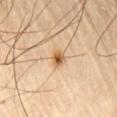Q: Is there a histopathology result?
A: imaged on a skin check; not biopsied
Q: What did automated image analysis measure?
A: an area of roughly 3 mm², an outline eccentricity of about 0.6 (0 = round, 1 = elongated), and a symmetry-axis asymmetry near 0.2
Q: What kind of image is this?
A: ~15 mm tile from a whole-body skin photo
Q: What are the patient's age and sex?
A: male, in their mid-60s
Q: Lesion size?
A: about 2 mm
Q: Where on the body is the lesion?
A: the chest
Q: What lighting was used for the tile?
A: cross-polarized